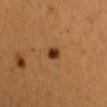This lesion was catalogued during total-body skin photography and was not selected for biopsy. The lesion's longest dimension is about 2 mm. The total-body-photography lesion software estimated a mean CIELAB color near L≈35 a*≈23 b*≈33 and a lesion–skin lightness drop of about 15. A close-up tile cropped from a whole-body skin photograph, about 15 mm across. A female patient, approximately 25 years of age. From the right forearm. Captured under cross-polarized illumination.